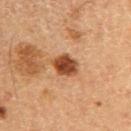{
  "biopsy_status": "not biopsied; imaged during a skin examination",
  "lesion_size": {
    "long_diameter_mm_approx": 3.5
  },
  "patient": {
    "sex": "male",
    "age_approx": 60
  },
  "automated_metrics": {
    "area_mm2_approx": 6.5,
    "eccentricity": 0.65,
    "shape_asymmetry": 0.2,
    "vs_skin_darker_L": 15.0,
    "vs_skin_contrast_norm": 11.5,
    "color_variation_0_10": 3.5,
    "peripheral_color_asymmetry": 1.0,
    "lesion_detection_confidence_0_100": 100
  },
  "image": {
    "source": "total-body photography crop",
    "field_of_view_mm": 15
  },
  "lighting": "cross-polarized"
}